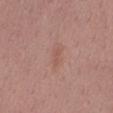The lesion was photographed on a routine skin check and not biopsied; there is no pathology result.
The lesion's longest dimension is about 2.5 mm.
Cropped from a whole-body photographic skin survey; the tile spans about 15 mm.
A female patient aged 48 to 52.
The lesion is on the back.
Imaged with white-light lighting.